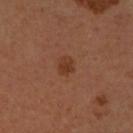Recorded during total-body skin imaging; not selected for excision or biopsy. The patient is a female aged 43–47. Located on the right forearm. Cropped from a total-body skin-imaging series; the visible field is about 15 mm.